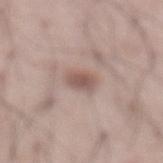Part of a total-body skin-imaging series; this lesion was reviewed on a skin check and was not flagged for biopsy. A male patient aged 53–57. The recorded lesion diameter is about 2.5 mm. The lesion is located on the abdomen. A roughly 15 mm field-of-view crop from a total-body skin photograph. The total-body-photography lesion software estimated roughly 11 lightness units darker than nearby skin and a lesion-to-skin contrast of about 7.5 (normalized; higher = more distinct).